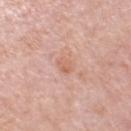Q: Was a biopsy performed?
A: total-body-photography surveillance lesion; no biopsy
Q: How was the tile lit?
A: white-light illumination
Q: Where on the body is the lesion?
A: the right upper arm
Q: Automated lesion metrics?
A: an automated nevus-likeness rating near 0 out of 100 and a lesion-detection confidence of about 100/100
Q: How was this image acquired?
A: total-body-photography crop, ~15 mm field of view
Q: What are the patient's age and sex?
A: female, about 50 years old
Q: How large is the lesion?
A: ≈2.5 mm